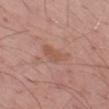<tbp_lesion>
<biopsy_status>not biopsied; imaged during a skin examination</biopsy_status>
<lesion_size>
  <long_diameter_mm_approx>4.0</long_diameter_mm_approx>
</lesion_size>
<lighting>white-light</lighting>
<patient>
  <sex>male</sex>
  <age_approx>55</age_approx>
</patient>
<automated_metrics>
  <area_mm2_approx>6.0</area_mm2_approx>
  <eccentricity>0.9</eccentricity>
  <lesion_detection_confidence_0_100>100</lesion_detection_confidence_0_100>
</automated_metrics>
<site>left thigh</site>
<image>
  <source>total-body photography crop</source>
  <field_of_view_mm>15</field_of_view_mm>
</image>
</tbp_lesion>